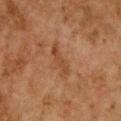The lesion was tiled from a total-body skin photograph and was not biopsied.
A female subject, aged approximately 60.
The lesion is located on the chest.
A lesion tile, about 15 mm wide, cut from a 3D total-body photograph.